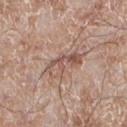biopsy_status: not biopsied; imaged during a skin examination
lighting: white-light
site: left lower leg
automated_metrics:
  area_mm2_approx: 8.0
  eccentricity: 0.9
  shape_asymmetry: 0.35
  cielab_L: 54
  cielab_a: 18
  cielab_b: 25
  vs_skin_darker_L: 9.0
  vs_skin_contrast_norm: 6.5
  border_irregularity_0_10: 5.0
  peripheral_color_asymmetry: 3.5
  nevus_likeness_0_100: 0
  lesion_detection_confidence_0_100: 90
lesion_size:
  long_diameter_mm_approx: 5.0
image:
  source: total-body photography crop
  field_of_view_mm: 15
patient:
  sex: male
  age_approx: 60